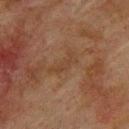notes: no biopsy performed (imaged during a skin exam) | TBP lesion metrics: a footprint of about 4.5 mm², an outline eccentricity of about 0.95 (0 = round, 1 = elongated), and two-axis asymmetry of about 0.5; a border-irregularity rating of about 7.5/10 and peripheral color asymmetry of about 0; a classifier nevus-likeness of about 0/100 and a detector confidence of about 95 out of 100 that the crop contains a lesion | anatomic site: the upper back | patient: male, about 75 years old | image: 15 mm crop, total-body photography | illumination: cross-polarized illumination.